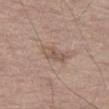Impression: Imaged during a routine full-body skin examination; the lesion was not biopsied and no histopathology is available. Clinical summary: A male patient, about 70 years old. The lesion's longest dimension is about 3.5 mm. Located on the left thigh. Captured under white-light illumination. A 15 mm close-up tile from a total-body photography series done for melanoma screening.